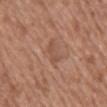| field | value |
|---|---|
| workup | total-body-photography surveillance lesion; no biopsy |
| automated metrics | a lesion color around L≈51 a*≈21 b*≈29 in CIELAB and a normalized border contrast of about 4.5; a color-variation rating of about 1/10 and a peripheral color-asymmetry measure near 0.5; an automated nevus-likeness rating near 0 out of 100 and a lesion-detection confidence of about 100/100 |
| anatomic site | the back |
| image source | ~15 mm tile from a whole-body skin photo |
| tile lighting | white-light |
| patient | female, in their mid- to late 70s |